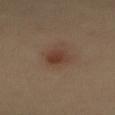Notes:
- biopsy status · total-body-photography surveillance lesion; no biopsy
- patient · male, aged 38 to 42
- size · ≈3.5 mm
- TBP lesion metrics · a lesion color around L≈37 a*≈18 b*≈26 in CIELAB, roughly 8 lightness units darker than nearby skin, and a normalized border contrast of about 7.5; a border-irregularity index near 1.5/10, a color-variation rating of about 3.5/10, and peripheral color asymmetry of about 1
- image · ~15 mm crop, total-body skin-cancer survey
- site · the abdomen